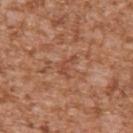Q: Was a biopsy performed?
A: catalogued during a skin exam; not biopsied
Q: Patient demographics?
A: male, approximately 45 years of age
Q: How large is the lesion?
A: about 3 mm
Q: Illumination type?
A: white-light
Q: How was this image acquired?
A: ~15 mm tile from a whole-body skin photo
Q: Lesion location?
A: the right upper arm